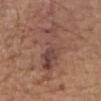Q: What lighting was used for the tile?
A: white-light illumination
Q: What is the anatomic site?
A: the front of the torso
Q: How large is the lesion?
A: ~8.5 mm (longest diameter)
Q: What are the patient's age and sex?
A: male, aged around 65
Q: What is the imaging modality?
A: total-body-photography crop, ~15 mm field of view